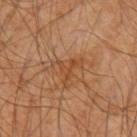Notes:
• notes: total-body-photography surveillance lesion; no biopsy
• image source: 15 mm crop, total-body photography
• site: the left upper arm
• illumination: cross-polarized
• patient: male, aged 58 to 62